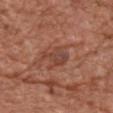Part of a total-body skin-imaging series; this lesion was reviewed on a skin check and was not flagged for biopsy. The lesion's longest dimension is about 3.5 mm. Located on the chest. Cropped from a whole-body photographic skin survey; the tile spans about 15 mm. This is a white-light tile. A female patient aged 73–77.